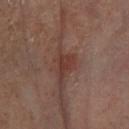workup — total-body-photography surveillance lesion; no biopsy
automated metrics — a footprint of about 3.5 mm², an outline eccentricity of about 0.85 (0 = round, 1 = elongated), and a shape-asymmetry score of about 0.55 (0 = symmetric); a border-irregularity rating of about 5.5/10, a within-lesion color-variation index near 1/10, and radial color variation of about 0.5
imaging modality — 15 mm crop, total-body photography
location — the left lower leg
subject — female, in their 50s